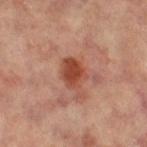| feature | finding |
|---|---|
| acquisition | ~15 mm crop, total-body skin-cancer survey |
| size | about 3.5 mm |
| automated metrics | a lesion area of about 7.5 mm², a shape eccentricity near 0.7, and a shape-asymmetry score of about 0.25 (0 = symmetric); about 11 CIELAB-L* units darker than the surrounding skin and a normalized border contrast of about 9 |
| subject | female, aged around 65 |
| location | the leg |